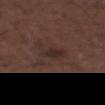Case summary:
* notes · imaged on a skin check; not biopsied
* acquisition · total-body-photography crop, ~15 mm field of view
* tile lighting · white-light
* lesion size · ≈4.5 mm
* anatomic site · the right forearm
* patient · male, in their 50s